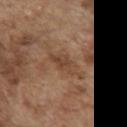workup = no biopsy performed (imaged during a skin exam) | body site = the front of the torso | acquisition = 15 mm crop, total-body photography | patient = male, aged approximately 75.